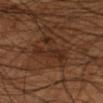The lesion was photographed on a routine skin check and not biopsied; there is no pathology result. Imaged with cross-polarized lighting. Cropped from a total-body skin-imaging series; the visible field is about 15 mm. A male subject in their mid-50s. Automated image analysis of the tile measured an outline eccentricity of about 0.85 (0 = round, 1 = elongated) and a shape-asymmetry score of about 0.5 (0 = symmetric). The software also gave roughly 6 lightness units darker than nearby skin and a lesion-to-skin contrast of about 7 (normalized; higher = more distinct). The analysis additionally found an automated nevus-likeness rating near 0 out of 100 and a lesion-detection confidence of about 75/100. Approximately 6 mm at its widest. From the right lower leg.